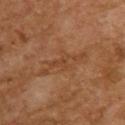| key | value |
|---|---|
| workup | no biopsy performed (imaged during a skin exam) |
| acquisition | ~15 mm tile from a whole-body skin photo |
| subject | female, aged around 60 |
| automated lesion analysis | an area of roughly 4.5 mm², an eccentricity of roughly 0.55, and a symmetry-axis asymmetry near 0.6; a mean CIELAB color near L≈37 a*≈19 b*≈30, about 5 CIELAB-L* units darker than the surrounding skin, and a normalized border contrast of about 4.5; a lesion-detection confidence of about 75/100 |
| tile lighting | cross-polarized |
| body site | the upper back |